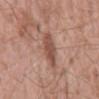Q: Was a biopsy performed?
A: no biopsy performed (imaged during a skin exam)
Q: What is the imaging modality?
A: ~15 mm crop, total-body skin-cancer survey
Q: What is the anatomic site?
A: the back
Q: What lighting was used for the tile?
A: white-light
Q: Lesion size?
A: ≈4 mm
Q: Who is the patient?
A: male, aged 58 to 62